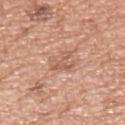follow-up: no biopsy performed (imaged during a skin exam); tile lighting: white-light; acquisition: ~15 mm tile from a whole-body skin photo; patient: male, in their mid- to late 60s; lesion size: about 3.5 mm; site: the left upper arm.